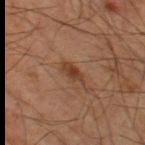workup: catalogued during a skin exam; not biopsied
location: the right thigh
image source: 15 mm crop, total-body photography
subject: male, aged approximately 60
TBP lesion metrics: an average lesion color of about L≈36 a*≈19 b*≈28 (CIELAB) and roughly 8 lightness units darker than nearby skin; a border-irregularity rating of about 3/10, a within-lesion color-variation index near 2.5/10, and radial color variation of about 0.5
lesion diameter: ~3.5 mm (longest diameter)
illumination: cross-polarized